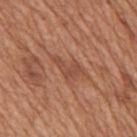| feature | finding |
|---|---|
| follow-up | imaged on a skin check; not biopsied |
| TBP lesion metrics | an outline eccentricity of about 0.85 (0 = round, 1 = elongated); a mean CIELAB color near L≈48 a*≈24 b*≈31, a lesion–skin lightness drop of about 7, and a normalized lesion–skin contrast near 5.5; a peripheral color-asymmetry measure near 1 |
| anatomic site | the mid back |
| tile lighting | white-light |
| lesion diameter | about 3.5 mm |
| subject | male, approximately 65 years of age |
| image source | total-body-photography crop, ~15 mm field of view |